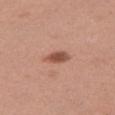body site = the right thigh
acquisition = total-body-photography crop, ~15 mm field of view
lighting = white-light
TBP lesion metrics = a lesion area of about 4.5 mm², a shape eccentricity near 0.8, and two-axis asymmetry of about 0.3; a mean CIELAB color near L≈52 a*≈24 b*≈29 and a normalized lesion–skin contrast near 9; a border-irregularity index near 3/10, internal color variation of about 3.5 on a 0–10 scale, and peripheral color asymmetry of about 1.5
lesion size = ~3 mm (longest diameter)
patient = female, in their 30s